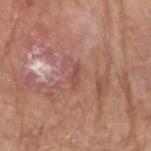body site: the right upper arm | illumination: white-light illumination | image-analysis metrics: an area of roughly 2 mm² and a shape eccentricity near 0.95 | image source: ~15 mm tile from a whole-body skin photo | patient: male, aged around 80.